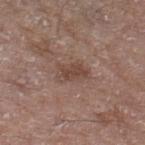Q: Was this lesion biopsied?
A: total-body-photography surveillance lesion; no biopsy